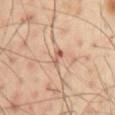Recorded during total-body skin imaging; not selected for excision or biopsy.
A 15 mm crop from a total-body photograph taken for skin-cancer surveillance.
This is a cross-polarized tile.
An algorithmic analysis of the crop reported an average lesion color of about L≈59 a*≈22 b*≈29 (CIELAB), a lesion–skin lightness drop of about 10, and a normalized lesion–skin contrast near 7. And it measured a classifier nevus-likeness of about 0/100 and lesion-presence confidence of about 100/100.
The lesion's longest dimension is about 2.5 mm.
The lesion is on the left arm.
The subject is a male approximately 50 years of age.